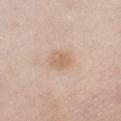Clinical impression: Part of a total-body skin-imaging series; this lesion was reviewed on a skin check and was not flagged for biopsy. Context: A male patient aged approximately 55. This is a white-light tile. On the front of the torso. A 15 mm close-up extracted from a 3D total-body photography capture.